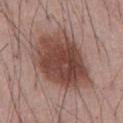Q: Is there a histopathology result?
A: catalogued during a skin exam; not biopsied
Q: How large is the lesion?
A: ~9 mm (longest diameter)
Q: What lighting was used for the tile?
A: white-light
Q: What is the anatomic site?
A: the abdomen
Q: What did automated image analysis measure?
A: a nevus-likeness score of about 85/100 and lesion-presence confidence of about 100/100
Q: What kind of image is this?
A: ~15 mm tile from a whole-body skin photo
Q: What are the patient's age and sex?
A: male, approximately 55 years of age